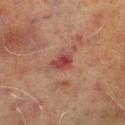Case summary:
– biopsy status — total-body-photography surveillance lesion; no biopsy
– anatomic site — the left lower leg
– tile lighting — cross-polarized illumination
– image source — ~15 mm crop, total-body skin-cancer survey
– subject — male, about 70 years old